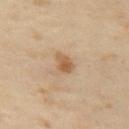Impression: The lesion was tiled from a total-body skin photograph and was not biopsied. Image and clinical context: A female patient approximately 50 years of age. A 15 mm crop from a total-body photograph taken for skin-cancer surveillance. Measured at roughly 2.5 mm in maximum diameter. Located on the leg.